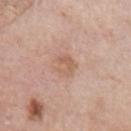– workup · imaged on a skin check; not biopsied
– image-analysis metrics · a lesion area of about 4.5 mm² and a shape eccentricity near 0.65; a lesion color around L≈60 a*≈20 b*≈30 in CIELAB, roughly 7 lightness units darker than nearby skin, and a lesion-to-skin contrast of about 5.5 (normalized; higher = more distinct); a nevus-likeness score of about 0/100 and lesion-presence confidence of about 100/100
– diameter · ~3 mm (longest diameter)
– illumination · white-light illumination
– patient · male, aged 73–77
– image source · ~15 mm tile from a whole-body skin photo
– location · the arm